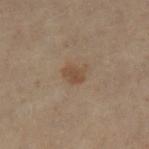The lesion was tiled from a total-body skin photograph and was not biopsied. About 2.5 mm across. Located on the left lower leg. The patient is a female aged around 50. A 15 mm close-up extracted from a 3D total-body photography capture. Imaged with cross-polarized lighting.